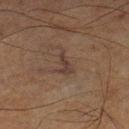Context: About 3.5 mm across. From the right lower leg. A male subject approximately 65 years of age. Captured under cross-polarized illumination. An algorithmic analysis of the crop reported a footprint of about 3.5 mm², an eccentricity of roughly 0.9, and two-axis asymmetry of about 0.45. It also reported a border-irregularity index near 5/10 and a color-variation rating of about 1/10. The analysis additionally found a lesion-detection confidence of about 90/100. A region of skin cropped from a whole-body photographic capture, roughly 15 mm wide.